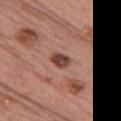follow-up = no biopsy performed (imaged during a skin exam) | patient = female, about 60 years old | anatomic site = the chest | tile lighting = white-light illumination | image source = ~15 mm tile from a whole-body skin photo | lesion size = ≈2.5 mm.